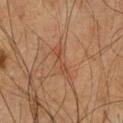{
  "biopsy_status": "not biopsied; imaged during a skin examination",
  "site": "chest",
  "patient": {
    "sex": "male",
    "age_approx": 65
  },
  "lesion_size": {
    "long_diameter_mm_approx": 4.5
  },
  "image": {
    "source": "total-body photography crop",
    "field_of_view_mm": 15
  },
  "automated_metrics": {
    "area_mm2_approx": 4.5,
    "shape_asymmetry": 0.4,
    "cielab_L": 39,
    "cielab_a": 20,
    "cielab_b": 27,
    "vs_skin_darker_L": 6.0,
    "vs_skin_contrast_norm": 5.0,
    "border_irregularity_0_10": 6.0,
    "color_variation_0_10": 0.5,
    "peripheral_color_asymmetry": 0.0,
    "nevus_likeness_0_100": 0,
    "lesion_detection_confidence_0_100": 95
  }
}